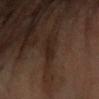workup: no biopsy performed (imaged during a skin exam); tile lighting: cross-polarized; image: ~15 mm tile from a whole-body skin photo; site: the left forearm; patient: female, aged 68–72; diameter: ~2.5 mm (longest diameter).